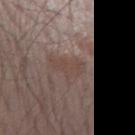Background: The patient is a male aged 53 to 57. A 15 mm close-up extracted from a 3D total-body photography capture. The lesion-visualizer software estimated a lesion area of about 5.5 mm² and two-axis asymmetry of about 0.35. The analysis additionally found a classifier nevus-likeness of about 0/100 and a detector confidence of about 95 out of 100 that the crop contains a lesion. The lesion is on the arm.